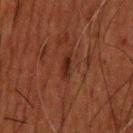Q: Was this lesion biopsied?
A: catalogued during a skin exam; not biopsied
Q: How was this image acquired?
A: ~15 mm crop, total-body skin-cancer survey
Q: What are the patient's age and sex?
A: male, aged approximately 50
Q: What is the lesion's diameter?
A: about 3 mm
Q: How was the tile lit?
A: cross-polarized illumination
Q: Automated lesion metrics?
A: an area of roughly 3 mm², a shape eccentricity near 0.9, and a shape-asymmetry score of about 0.25 (0 = symmetric); a mean CIELAB color near L≈21 a*≈20 b*≈23, about 6 CIELAB-L* units darker than the surrounding skin, and a lesion-to-skin contrast of about 7.5 (normalized; higher = more distinct); peripheral color asymmetry of about 1; lesion-presence confidence of about 95/100
Q: Where on the body is the lesion?
A: the head or neck